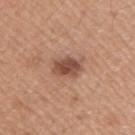| field | value |
|---|---|
| acquisition | 15 mm crop, total-body photography |
| location | the right upper arm |
| patient | male, aged approximately 20 |